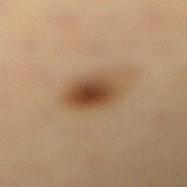This lesion was catalogued during total-body skin photography and was not selected for biopsy. The lesion is on the leg. A female subject, aged around 40. The lesion's longest dimension is about 4.5 mm. A 15 mm close-up tile from a total-body photography series done for melanoma screening.